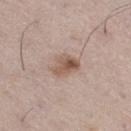| key | value |
|---|---|
| follow-up | no biopsy performed (imaged during a skin exam) |
| automated metrics | a lesion area of about 6.5 mm², a shape eccentricity near 0.75, and a shape-asymmetry score of about 0.25 (0 = symmetric); an average lesion color of about L≈55 a*≈17 b*≈26 (CIELAB), about 11 CIELAB-L* units darker than the surrounding skin, and a normalized lesion–skin contrast near 8; a nevus-likeness score of about 80/100 and lesion-presence confidence of about 100/100 |
| anatomic site | the right thigh |
| patient | male, about 55 years old |
| lesion size | ≈3.5 mm |
| acquisition | ~15 mm crop, total-body skin-cancer survey |
| tile lighting | white-light |